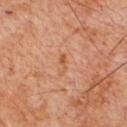Findings:
– biopsy status — imaged on a skin check; not biopsied
– patient — male, about 60 years old
– image-analysis metrics — a lesion area of about 2 mm², an outline eccentricity of about 0.95 (0 = round, 1 = elongated), and a symmetry-axis asymmetry near 0.45; a lesion color around L≈54 a*≈24 b*≈37 in CIELAB, about 7 CIELAB-L* units darker than the surrounding skin, and a lesion-to-skin contrast of about 6 (normalized; higher = more distinct); a border-irregularity index near 5.5/10, internal color variation of about 0 on a 0–10 scale, and a peripheral color-asymmetry measure near 0
– acquisition — ~15 mm crop, total-body skin-cancer survey
– lesion diameter — about 2.5 mm
– site — the chest